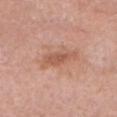The lesion was tiled from a total-body skin photograph and was not biopsied.
A female subject, in their mid- to late 80s or older.
The lesion is on the head or neck.
Cropped from a whole-body photographic skin survey; the tile spans about 15 mm.